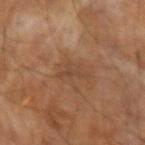The subject is a male in their mid- to late 60s. Cropped from a total-body skin-imaging series; the visible field is about 15 mm. Located on the arm. This is a cross-polarized tile. The total-body-photography lesion software estimated a lesion color around L≈43 a*≈19 b*≈29 in CIELAB, about 6 CIELAB-L* units darker than the surrounding skin, and a lesion-to-skin contrast of about 5 (normalized; higher = more distinct). The analysis additionally found border irregularity of about 6.5 on a 0–10 scale and peripheral color asymmetry of about 0.5. The analysis additionally found a classifier nevus-likeness of about 0/100 and a lesion-detection confidence of about 100/100.